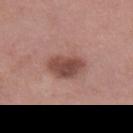Assessment:
Recorded during total-body skin imaging; not selected for excision or biopsy.
Background:
Cropped from a total-body skin-imaging series; the visible field is about 15 mm. Automated tile analysis of the lesion measured an average lesion color of about L≈47 a*≈22 b*≈24 (CIELAB), about 13 CIELAB-L* units darker than the surrounding skin, and a normalized lesion–skin contrast near 9.5. The analysis additionally found a border-irregularity index near 2/10 and a within-lesion color-variation index near 3.5/10. The patient is a female approximately 50 years of age. The tile uses white-light illumination. The lesion is located on the upper back.